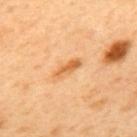{
  "biopsy_status": "not biopsied; imaged during a skin examination",
  "lesion_size": {
    "long_diameter_mm_approx": 3.5
  },
  "site": "mid back",
  "image": {
    "source": "total-body photography crop",
    "field_of_view_mm": 15
  },
  "patient": {
    "sex": "male",
    "age_approx": 50
  },
  "lighting": "cross-polarized",
  "automated_metrics": {
    "border_irregularity_0_10": 3.5,
    "color_variation_0_10": 1.5,
    "peripheral_color_asymmetry": 0.5,
    "nevus_likeness_0_100": 60,
    "lesion_detection_confidence_0_100": 100
  }
}